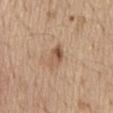Acquisition and patient details:
A male patient, aged approximately 70. Imaged with white-light lighting. The total-body-photography lesion software estimated an average lesion color of about L≈55 a*≈18 b*≈32 (CIELAB), roughly 10 lightness units darker than nearby skin, and a normalized lesion–skin contrast near 7. The lesion is on the abdomen. This image is a 15 mm lesion crop taken from a total-body photograph.